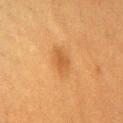follow-up: imaged on a skin check; not biopsied | image: ~15 mm tile from a whole-body skin photo | size: ~2.5 mm (longest diameter) | subject: female, aged 38–42 | site: the chest | lighting: cross-polarized.